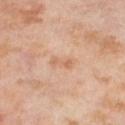| key | value |
|---|---|
| workup | catalogued during a skin exam; not biopsied |
| location | the right lower leg |
| TBP lesion metrics | lesion-presence confidence of about 100/100 |
| lighting | cross-polarized illumination |
| acquisition | ~15 mm crop, total-body skin-cancer survey |
| subject | female, aged approximately 55 |